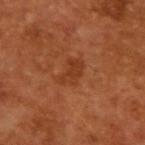This lesion was catalogued during total-body skin photography and was not selected for biopsy. Cropped from a total-body skin-imaging series; the visible field is about 15 mm. A male patient aged 63 to 67.